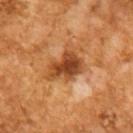No biopsy was performed on this lesion — it was imaged during a full skin examination and was not determined to be concerning.
Captured under cross-polarized illumination.
A 15 mm close-up extracted from a 3D total-body photography capture.
A male patient approximately 65 years of age.
Approximately 5 mm at its widest.
Automated tile analysis of the lesion measured an area of roughly 12 mm² and an eccentricity of roughly 0.7. The software also gave a mean CIELAB color near L≈46 a*≈26 b*≈39 and about 13 CIELAB-L* units darker than the surrounding skin. The analysis additionally found a border-irregularity index near 3.5/10, a within-lesion color-variation index near 6/10, and radial color variation of about 1.5. And it measured a nevus-likeness score of about 25/100 and a lesion-detection confidence of about 100/100.